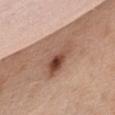notes: no biopsy performed (imaged during a skin exam) | anatomic site: the chest | diameter: about 6.5 mm | acquisition: ~15 mm crop, total-body skin-cancer survey | lighting: white-light illumination | patient: female, roughly 40 years of age.